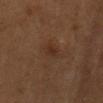patient:
  sex: male
  age_approx: 55
lighting: cross-polarized
image:
  source: total-body photography crop
  field_of_view_mm: 15
lesion_size:
  long_diameter_mm_approx: 3.0
site: chest